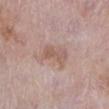Findings:
• image source — ~15 mm tile from a whole-body skin photo
• image-analysis metrics — a lesion area of about 6 mm² and a shape eccentricity near 0.9; an average lesion color of about L≈57 a*≈18 b*≈24 (CIELAB), roughly 8 lightness units darker than nearby skin, and a normalized lesion–skin contrast near 6; a nevus-likeness score of about 0/100 and a detector confidence of about 100 out of 100 that the crop contains a lesion
• diameter — ≈4 mm
• site — the leg
• patient — female, aged approximately 70